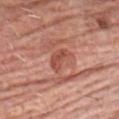workup: imaged on a skin check; not biopsied | body site: the chest | size: about 3 mm | tile lighting: white-light | subject: male, aged 78–82 | image: ~15 mm crop, total-body skin-cancer survey.